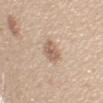This is a white-light tile. Located on the left upper arm. The total-body-photography lesion software estimated a lesion area of about 5.5 mm², an outline eccentricity of about 0.6 (0 = round, 1 = elongated), and two-axis asymmetry of about 0.25. The analysis additionally found a mean CIELAB color near L≈61 a*≈17 b*≈28, a lesion–skin lightness drop of about 11, and a normalized lesion–skin contrast near 7. The analysis additionally found a color-variation rating of about 4/10 and a peripheral color-asymmetry measure near 1.5. A female patient aged approximately 25. Cropped from a total-body skin-imaging series; the visible field is about 15 mm.